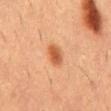| field | value |
|---|---|
| workup | catalogued during a skin exam; not biopsied |
| illumination | cross-polarized |
| image source | total-body-photography crop, ~15 mm field of view |
| location | the mid back |
| automated lesion analysis | an average lesion color of about L≈49 a*≈25 b*≈36 (CIELAB), about 11 CIELAB-L* units darker than the surrounding skin, and a lesion-to-skin contrast of about 8.5 (normalized; higher = more distinct); border irregularity of about 1.5 on a 0–10 scale, internal color variation of about 3 on a 0–10 scale, and a peripheral color-asymmetry measure near 1 |
| patient | male, approximately 55 years of age |